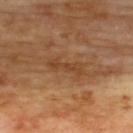location=the back | subject=male, aged 68–72 | image source=~15 mm tile from a whole-body skin photo.